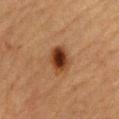No biopsy was performed on this lesion — it was imaged during a full skin examination and was not determined to be concerning. The lesion-visualizer software estimated an average lesion color of about L≈34 a*≈21 b*≈31 (CIELAB) and about 14 CIELAB-L* units darker than the surrounding skin. The analysis additionally found border irregularity of about 1.5 on a 0–10 scale, a color-variation rating of about 6.5/10, and radial color variation of about 2. The analysis additionally found a nevus-likeness score of about 100/100. A 15 mm crop from a total-body photograph taken for skin-cancer surveillance. The lesion is located on the abdomen. A male patient, aged 83 or older. Imaged with cross-polarized lighting. Approximately 3.5 mm at its widest.